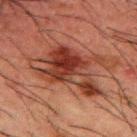Impression:
Part of a total-body skin-imaging series; this lesion was reviewed on a skin check and was not flagged for biopsy.
Clinical summary:
The patient is a male approximately 50 years of age. On the back. Automated tile analysis of the lesion measured a border-irregularity index near 7/10, a within-lesion color-variation index near 9/10, and radial color variation of about 3.5. Captured under cross-polarized illumination. A region of skin cropped from a whole-body photographic capture, roughly 15 mm wide.